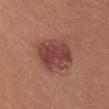Impression:
The lesion was tiled from a total-body skin photograph and was not biopsied.
Background:
A 15 mm close-up tile from a total-body photography series done for melanoma screening. Automated image analysis of the tile measured an average lesion color of about L≈43 a*≈25 b*≈25 (CIELAB), roughly 11 lightness units darker than nearby skin, and a normalized border contrast of about 8.5. It also reported a border-irregularity index near 2.5/10 and peripheral color asymmetry of about 1.5. The analysis additionally found lesion-presence confidence of about 100/100. The tile uses white-light illumination. The lesion is located on the upper back. A female patient aged 33 to 37. Measured at roughly 5 mm in maximum diameter.